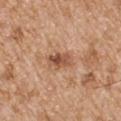| field | value |
|---|---|
| patient | male, approximately 65 years of age |
| lighting | white-light |
| image | 15 mm crop, total-body photography |
| lesion size | ≈3 mm |
| site | the left upper arm |
| automated metrics | a lesion area of about 5.5 mm², an eccentricity of roughly 0.8, and a shape-asymmetry score of about 0.2 (0 = symmetric); a lesion color around L≈52 a*≈23 b*≈33 in CIELAB and a normalized border contrast of about 8; border irregularity of about 2.5 on a 0–10 scale, a color-variation rating of about 6.5/10, and peripheral color asymmetry of about 2.5; a nevus-likeness score of about 0/100 |